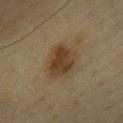The lesion was tiled from a total-body skin photograph and was not biopsied. The subject is a female aged 58–62. On the chest. A 15 mm crop from a total-body photograph taken for skin-cancer surveillance.